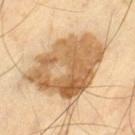body site=the left thigh; subject=male, aged 53–57; image=15 mm crop, total-body photography.